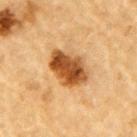No biopsy was performed on this lesion — it was imaged during a full skin examination and was not determined to be concerning.
From the arm.
The lesion's longest dimension is about 5 mm.
The subject is a male aged 83 or older.
This is a cross-polarized tile.
A region of skin cropped from a whole-body photographic capture, roughly 15 mm wide.